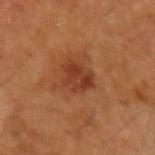site: the left upper arm; tile lighting: cross-polarized; patient: male, about 60 years old; image: total-body-photography crop, ~15 mm field of view.